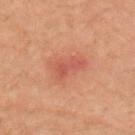workup — imaged on a skin check; not biopsied
subject — female, aged around 60
site — the right upper arm
image source — total-body-photography crop, ~15 mm field of view
lesion size — about 3.5 mm